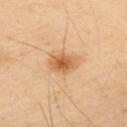Acquisition and patient details:
Captured under cross-polarized illumination. The lesion's longest dimension is about 4 mm. An algorithmic analysis of the crop reported a footprint of about 8 mm² and an outline eccentricity of about 0.75 (0 = round, 1 = elongated). It also reported border irregularity of about 1.5 on a 0–10 scale, a within-lesion color-variation index near 5/10, and radial color variation of about 1. The analysis additionally found a classifier nevus-likeness of about 95/100 and a lesion-detection confidence of about 100/100. The patient is a male aged approximately 50. The lesion is on the upper back. This image is a 15 mm lesion crop taken from a total-body photograph.